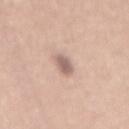  biopsy_status: not biopsied; imaged during a skin examination
  patient:
    sex: female
    age_approx: 45
  image:
    source: total-body photography crop
    field_of_view_mm: 15
  site: mid back